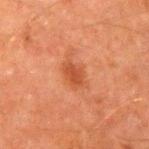Clinical impression:
Imaged during a routine full-body skin examination; the lesion was not biopsied and no histopathology is available.
Clinical summary:
Located on the right upper arm. A 15 mm crop from a total-body photograph taken for skin-cancer surveillance. A male subject, in their 60s.